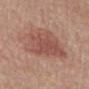{"biopsy_status": "not biopsied; imaged during a skin examination", "patient": {"sex": "male", "age_approx": 80}, "image": {"source": "total-body photography crop", "field_of_view_mm": 15}, "automated_metrics": {"eccentricity": 0.8, "shape_asymmetry": 0.25, "border_irregularity_0_10": 3.0, "color_variation_0_10": 4.0, "peripheral_color_asymmetry": 1.0}, "site": "mid back"}